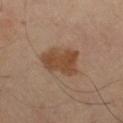No biopsy was performed on this lesion — it was imaged during a full skin examination and was not determined to be concerning. The recorded lesion diameter is about 5 mm. This image is a 15 mm lesion crop taken from a total-body photograph. This is a cross-polarized tile. A male subject, approximately 65 years of age. On the right thigh.